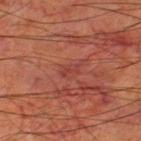On the left thigh. Imaged with cross-polarized lighting. The subject is a male aged approximately 80. The lesion's longest dimension is about 3 mm. A 15 mm close-up tile from a total-body photography series done for melanoma screening.